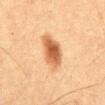biopsy_status: not biopsied; imaged during a skin examination
patient:
  sex: male
  age_approx: 50
automated_metrics:
  area_mm2_approx: 11.0
  eccentricity: 0.8
  shape_asymmetry: 0.2
  nevus_likeness_0_100: 100
  lesion_detection_confidence_0_100: 100
lesion_size:
  long_diameter_mm_approx: 5.0
site: abdomen
image:
  source: total-body photography crop
  field_of_view_mm: 15